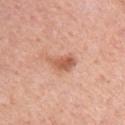| key | value |
|---|---|
| biopsy status | imaged on a skin check; not biopsied |
| location | the arm |
| size | ~3.5 mm (longest diameter) |
| image source | 15 mm crop, total-body photography |
| lighting | white-light illumination |
| subject | female, aged around 60 |